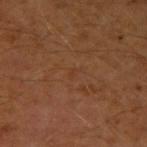Imaged during a routine full-body skin examination; the lesion was not biopsied and no histopathology is available. Located on the right upper arm. The subject is a male roughly 60 years of age. A 15 mm close-up tile from a total-body photography series done for melanoma screening. The tile uses cross-polarized illumination.